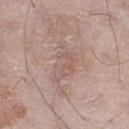Case summary:
• workup — no biopsy performed (imaged during a skin exam)